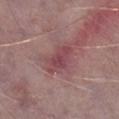Impression:
Imaged during a routine full-body skin examination; the lesion was not biopsied and no histopathology is available.
Acquisition and patient details:
A male subject aged approximately 75. The tile uses white-light illumination. A lesion tile, about 15 mm wide, cut from a 3D total-body photograph. Automated tile analysis of the lesion measured a border-irregularity index near 3.5/10, a color-variation rating of about 2.5/10, and peripheral color asymmetry of about 1. The software also gave a lesion-detection confidence of about 95/100. Measured at roughly 4.5 mm in maximum diameter. The lesion is located on the right lower leg.